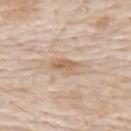patient: male, aged 73–77 | image: ~15 mm crop, total-body skin-cancer survey | size: ≈3 mm | site: the upper back | illumination: white-light illumination | TBP lesion metrics: an eccentricity of roughly 0.85; a border-irregularity index near 3.5/10 and peripheral color asymmetry of about 1.